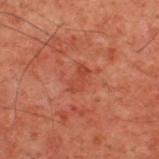Q: How was this image acquired?
A: 15 mm crop, total-body photography
Q: Who is the patient?
A: male, aged approximately 60
Q: Illumination type?
A: cross-polarized illumination
Q: Where on the body is the lesion?
A: the upper back
Q: What did automated image analysis measure?
A: a lesion–skin lightness drop of about 5 and a lesion-to-skin contrast of about 5 (normalized; higher = more distinct); a classifier nevus-likeness of about 0/100 and lesion-presence confidence of about 100/100
Q: Lesion size?
A: ~3 mm (longest diameter)